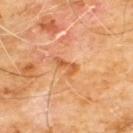Notes:
* biopsy status: catalogued during a skin exam; not biopsied
* subject: male, about 60 years old
* lesion diameter: ~3 mm (longest diameter)
* illumination: cross-polarized illumination
* anatomic site: the chest
* acquisition: 15 mm crop, total-body photography